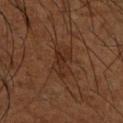Imaged during a routine full-body skin examination; the lesion was not biopsied and no histopathology is available. Automated tile analysis of the lesion measured a lesion area of about 6 mm² and an eccentricity of roughly 0.85. The analysis additionally found border irregularity of about 5.5 on a 0–10 scale, internal color variation of about 2 on a 0–10 scale, and a peripheral color-asymmetry measure near 0.5. Cropped from a total-body skin-imaging series; the visible field is about 15 mm. From the leg. The subject is a male aged 63 to 67. The tile uses cross-polarized illumination. About 4 mm across.